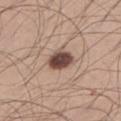This lesion was catalogued during total-body skin photography and was not selected for biopsy. On the right thigh. A lesion tile, about 15 mm wide, cut from a 3D total-body photograph. A male subject aged 23 to 27.